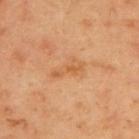This lesion was catalogued during total-body skin photography and was not selected for biopsy. The lesion is on the back. Automated tile analysis of the lesion measured an average lesion color of about L≈59 a*≈24 b*≈41 (CIELAB), a lesion–skin lightness drop of about 7, and a normalized border contrast of about 5. A lesion tile, about 15 mm wide, cut from a 3D total-body photograph. This is a cross-polarized tile. The recorded lesion diameter is about 4 mm. A male subject aged 43 to 47.